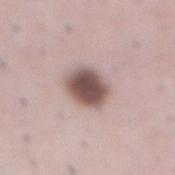Findings:
• biopsy status — catalogued during a skin exam; not biopsied
• anatomic site — the abdomen
• imaging modality — ~15 mm crop, total-body skin-cancer survey
• automated metrics — a mean CIELAB color near L≈52 a*≈17 b*≈19 and about 18 CIELAB-L* units darker than the surrounding skin
• illumination — white-light
• patient — male, approximately 50 years of age
• lesion diameter — ≈4 mm